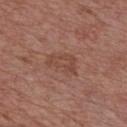Case summary:
- workup — no biopsy performed (imaged during a skin exam)
- TBP lesion metrics — a footprint of about 7.5 mm², a shape eccentricity near 0.8, and a symmetry-axis asymmetry near 0.35; internal color variation of about 2.5 on a 0–10 scale and radial color variation of about 1
- body site — the chest
- lesion diameter — ~4 mm (longest diameter)
- imaging modality — 15 mm crop, total-body photography
- subject — male, about 70 years old
- lighting — white-light